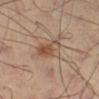biopsy_status: not biopsied; imaged during a skin examination